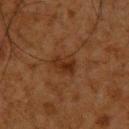Assessment:
Recorded during total-body skin imaging; not selected for excision or biopsy.
Clinical summary:
Longest diameter approximately 3 mm. The total-body-photography lesion software estimated a shape-asymmetry score of about 0.25 (0 = symmetric). The software also gave a lesion color around L≈25 a*≈19 b*≈28 in CIELAB, roughly 6 lightness units darker than nearby skin, and a normalized lesion–skin contrast near 7. A 15 mm crop from a total-body photograph taken for skin-cancer surveillance. Imaged with cross-polarized lighting. The patient is a male aged 58 to 62. Located on the upper back.